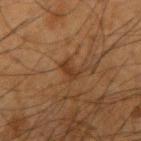Q: Was a biopsy performed?
A: no biopsy performed (imaged during a skin exam)
Q: Automated lesion metrics?
A: an area of roughly 4 mm² and a symmetry-axis asymmetry near 0.5; a lesion color around L≈30 a*≈17 b*≈27 in CIELAB, roughly 6 lightness units darker than nearby skin, and a normalized lesion–skin contrast near 6.5; a border-irregularity rating of about 5.5/10, a within-lesion color-variation index near 2/10, and a peripheral color-asymmetry measure near 0.5; an automated nevus-likeness rating near 0 out of 100
Q: Lesion size?
A: about 3 mm
Q: What kind of image is this?
A: ~15 mm tile from a whole-body skin photo
Q: Patient demographics?
A: male, aged approximately 65
Q: Where on the body is the lesion?
A: the right upper arm
Q: What lighting was used for the tile?
A: cross-polarized illumination